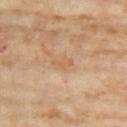workup=catalogued during a skin exam; not biopsied | automated metrics=border irregularity of about 3.5 on a 0–10 scale and radial color variation of about 0; a detector confidence of about 100 out of 100 that the crop contains a lesion | lesion size=≈2.5 mm | body site=the chest | subject=female, in their mid- to late 70s | lighting=cross-polarized | image source=total-body-photography crop, ~15 mm field of view.